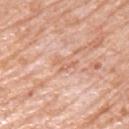biopsy status: catalogued during a skin exam; not biopsied | illumination: white-light | body site: the left upper arm | patient: male, aged around 80 | acquisition: 15 mm crop, total-body photography.